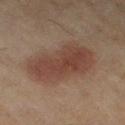Assessment: Part of a total-body skin-imaging series; this lesion was reviewed on a skin check and was not flagged for biopsy. Background: The patient is a female aged approximately 60. A roughly 15 mm field-of-view crop from a total-body skin photograph. Imaged with cross-polarized lighting. The lesion is located on the left thigh.